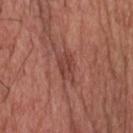notes: catalogued during a skin exam; not biopsied | patient: male, in their 60s | size: ~3.5 mm (longest diameter) | tile lighting: white-light | image: ~15 mm crop, total-body skin-cancer survey | site: the head or neck.